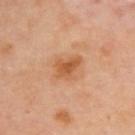biopsy status: total-body-photography surveillance lesion; no biopsy | location: the upper back | illumination: cross-polarized | patient: female, approximately 40 years of age | imaging modality: ~15 mm crop, total-body skin-cancer survey | TBP lesion metrics: a lesion area of about 6 mm² and a shape eccentricity near 0.65; a border-irregularity index near 4/10, a within-lesion color-variation index near 2.5/10, and a peripheral color-asymmetry measure near 0.5; a nevus-likeness score of about 50/100 | diameter: ~3 mm (longest diameter).